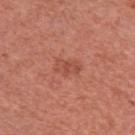follow-up = no biopsy performed (imaged during a skin exam).